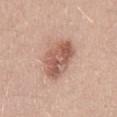Findings:
• notes: total-body-photography surveillance lesion; no biopsy
• anatomic site: the mid back
• illumination: white-light
• image: ~15 mm tile from a whole-body skin photo
• patient: male, aged approximately 35
• diameter: ~5.5 mm (longest diameter)